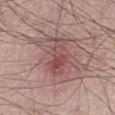* workup — catalogued during a skin exam; not biopsied
* anatomic site — the right lower leg
* imaging modality — ~15 mm tile from a whole-body skin photo
* patient — male, in their mid- to late 50s